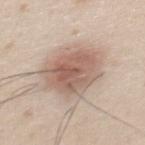Impression:
Part of a total-body skin-imaging series; this lesion was reviewed on a skin check and was not flagged for biopsy.
Clinical summary:
Located on the back. Captured under white-light illumination. The total-body-photography lesion software estimated a mean CIELAB color near L≈60 a*≈18 b*≈25, roughly 12 lightness units darker than nearby skin, and a normalized lesion–skin contrast near 8. And it measured a classifier nevus-likeness of about 90/100 and a detector confidence of about 100 out of 100 that the crop contains a lesion. Approximately 6 mm at its widest. A close-up tile cropped from a whole-body skin photograph, about 15 mm across. A male subject aged 23–27.